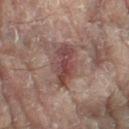follow-up — no biopsy performed (imaged during a skin exam) | lesion size — about 4.5 mm | body site — the right leg | image — 15 mm crop, total-body photography | subject — female, approximately 80 years of age.